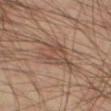* anatomic site · the left thigh
* illumination · cross-polarized
* lesion size · about 4.5 mm
* image · total-body-photography crop, ~15 mm field of view
* patient · male, aged 58–62
* automated lesion analysis · a footprint of about 11 mm² and a symmetry-axis asymmetry near 0.4; a mean CIELAB color near L≈47 a*≈17 b*≈26, about 8 CIELAB-L* units darker than the surrounding skin, and a normalized lesion–skin contrast near 6; a border-irregularity index near 4/10 and peripheral color asymmetry of about 1.5; a classifier nevus-likeness of about 5/100 and a lesion-detection confidence of about 60/100